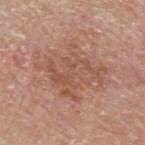site: upper back
image:
  source: total-body photography crop
  field_of_view_mm: 15
lesion_size:
  long_diameter_mm_approx: 7.0
lighting: white-light
patient:
  sex: male
  age_approx: 80